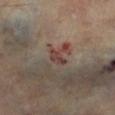notes: total-body-photography surveillance lesion; no biopsy | subject: female, aged approximately 60 | image-analysis metrics: an area of roughly 3 mm², an outline eccentricity of about 0.9 (0 = round, 1 = elongated), and a symmetry-axis asymmetry near 0.45; a lesion color around L≈39 a*≈19 b*≈21 in CIELAB and a lesion–skin lightness drop of about 8; an automated nevus-likeness rating near 0 out of 100 | body site: the leg | illumination: cross-polarized illumination | lesion size: about 3 mm | image source: total-body-photography crop, ~15 mm field of view.